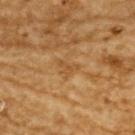Q: Was a biopsy performed?
A: imaged on a skin check; not biopsied
Q: What are the patient's age and sex?
A: male, in their mid- to late 80s or older
Q: What is the anatomic site?
A: the upper back
Q: What kind of image is this?
A: ~15 mm tile from a whole-body skin photo